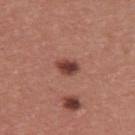The lesion was tiled from a total-body skin photograph and was not biopsied. From the upper back. A male patient about 40 years old. A lesion tile, about 15 mm wide, cut from a 3D total-body photograph.